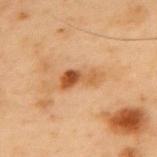follow-up=imaged on a skin check; not biopsied
body site=the upper back
imaging modality=~15 mm crop, total-body skin-cancer survey
illumination=cross-polarized illumination
automated lesion analysis=an area of roughly 7 mm²; border irregularity of about 4 on a 0–10 scale, a color-variation rating of about 9.5/10, and a peripheral color-asymmetry measure near 3.5; a classifier nevus-likeness of about 20/100 and lesion-presence confidence of about 100/100
diameter=about 4.5 mm
subject=male, aged 53 to 57